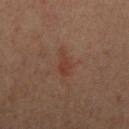A 15 mm close-up extracted from a 3D total-body photography capture.
The patient is a female aged approximately 45.
The lesion is on the arm.
Captured under cross-polarized illumination.
Automated tile analysis of the lesion measured border irregularity of about 4 on a 0–10 scale and a peripheral color-asymmetry measure near 0.5.